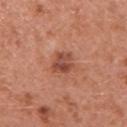Impression:
Imaged during a routine full-body skin examination; the lesion was not biopsied and no histopathology is available.
Acquisition and patient details:
A 15 mm close-up tile from a total-body photography series done for melanoma screening. The lesion is on the right upper arm. Measured at roughly 3 mm in maximum diameter. A female subject, approximately 40 years of age.